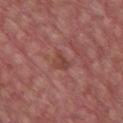Imaged during a routine full-body skin examination; the lesion was not biopsied and no histopathology is available.